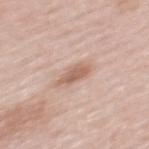Captured during whole-body skin photography for melanoma surveillance; the lesion was not biopsied. A female patient aged around 60. The lesion-visualizer software estimated a footprint of about 4.5 mm² and a symmetry-axis asymmetry near 0.25. The software also gave an automated nevus-likeness rating near 25 out of 100 and a detector confidence of about 100 out of 100 that the crop contains a lesion. Located on the upper back. A roughly 15 mm field-of-view crop from a total-body skin photograph.